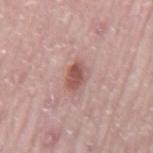Assessment:
Imaged during a routine full-body skin examination; the lesion was not biopsied and no histopathology is available.
Acquisition and patient details:
This image is a 15 mm lesion crop taken from a total-body photograph. The lesion is located on the back. A male subject, aged 73–77. The recorded lesion diameter is about 3.5 mm. Imaged with white-light lighting.